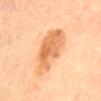This lesion was catalogued during total-body skin photography and was not selected for biopsy. An algorithmic analysis of the crop reported a lesion area of about 20 mm², an eccentricity of roughly 0.95, and a symmetry-axis asymmetry near 0.2. The analysis additionally found a border-irregularity index near 3.5/10 and radial color variation of about 1.5. A female patient aged 63 to 67. Measured at roughly 8.5 mm in maximum diameter. Cropped from a whole-body photographic skin survey; the tile spans about 15 mm. The lesion is on the back.